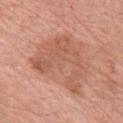<record>
<biopsy_status>not biopsied; imaged during a skin examination</biopsy_status>
<patient>
  <sex>male</sex>
  <age_approx>75</age_approx>
</patient>
<site>chest</site>
<lighting>white-light</lighting>
<lesion_size>
  <long_diameter_mm_approx>7.5</long_diameter_mm_approx>
</lesion_size>
<automated_metrics>
  <area_mm2_approx>22.0</area_mm2_approx>
  <shape_asymmetry>0.7</shape_asymmetry>
  <vs_skin_darker_L>8.0</vs_skin_darker_L>
  <vs_skin_contrast_norm>6.0</vs_skin_contrast_norm>
  <border_irregularity_0_10>10.0</border_irregularity_0_10>
  <peripheral_color_asymmetry>1.0</peripheral_color_asymmetry>
  <nevus_likeness_0_100>0</nevus_likeness_0_100>
</automated_metrics>
<image>
  <source>total-body photography crop</source>
  <field_of_view_mm>15</field_of_view_mm>
</image>
</record>